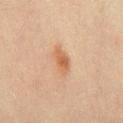Q: Was this lesion biopsied?
A: imaged on a skin check; not biopsied
Q: What are the patient's age and sex?
A: male, aged 43–47
Q: Lesion location?
A: the abdomen
Q: How large is the lesion?
A: ~3.5 mm (longest diameter)
Q: What lighting was used for the tile?
A: cross-polarized
Q: What is the imaging modality?
A: 15 mm crop, total-body photography